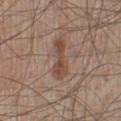follow-up: catalogued during a skin exam; not biopsied | site: the left lower leg | image: ~15 mm tile from a whole-body skin photo | patient: male, aged 58 to 62.